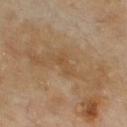Q: Was this lesion biopsied?
A: catalogued during a skin exam; not biopsied
Q: Where on the body is the lesion?
A: the chest
Q: Automated lesion metrics?
A: a within-lesion color-variation index near 0.5/10; a classifier nevus-likeness of about 0/100
Q: What is the imaging modality?
A: total-body-photography crop, ~15 mm field of view
Q: Patient demographics?
A: male, aged approximately 70